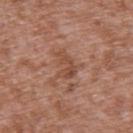Assessment:
The lesion was photographed on a routine skin check and not biopsied; there is no pathology result.
Acquisition and patient details:
Longest diameter approximately 3 mm. A close-up tile cropped from a whole-body skin photograph, about 15 mm across. The patient is a male aged 43–47. On the upper back. Captured under white-light illumination. The total-body-photography lesion software estimated a footprint of about 5.5 mm² and a shape-asymmetry score of about 0.4 (0 = symmetric). The software also gave a mean CIELAB color near L≈48 a*≈23 b*≈30 and about 8 CIELAB-L* units darker than the surrounding skin. And it measured border irregularity of about 5 on a 0–10 scale, internal color variation of about 3 on a 0–10 scale, and peripheral color asymmetry of about 1. The software also gave a classifier nevus-likeness of about 0/100 and a detector confidence of about 100 out of 100 that the crop contains a lesion.